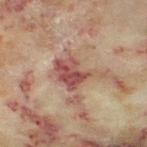Q: Was this lesion biopsied?
A: total-body-photography surveillance lesion; no biopsy
Q: Illumination type?
A: cross-polarized illumination
Q: Who is the patient?
A: female, aged around 60
Q: What kind of image is this?
A: total-body-photography crop, ~15 mm field of view
Q: How large is the lesion?
A: ~5.5 mm (longest diameter)
Q: What did automated image analysis measure?
A: a footprint of about 11 mm² and an outline eccentricity of about 0.75 (0 = round, 1 = elongated); a nevus-likeness score of about 0/100
Q: Lesion location?
A: the leg